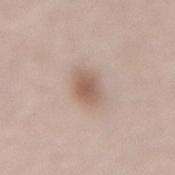Q: Is there a histopathology result?
A: catalogued during a skin exam; not biopsied
Q: What is the imaging modality?
A: ~15 mm crop, total-body skin-cancer survey
Q: Lesion location?
A: the lower back
Q: Illumination type?
A: white-light illumination
Q: Who is the patient?
A: female, about 65 years old
Q: What is the lesion's diameter?
A: about 3 mm